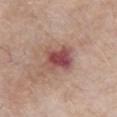Q: Is there a histopathology result?
A: no biopsy performed (imaged during a skin exam)
Q: Lesion size?
A: ≈3.5 mm
Q: What is the anatomic site?
A: the chest
Q: Automated lesion metrics?
A: a footprint of about 9.5 mm², an eccentricity of roughly 0.6, and a symmetry-axis asymmetry near 0.15; a nevus-likeness score of about 0/100
Q: What are the patient's age and sex?
A: male, approximately 70 years of age
Q: How was this image acquired?
A: ~15 mm tile from a whole-body skin photo